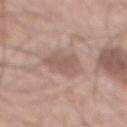A close-up tile cropped from a whole-body skin photograph, about 15 mm across. An algorithmic analysis of the crop reported a footprint of about 9.5 mm², a shape eccentricity near 0.5, and a symmetry-axis asymmetry near 0.2. And it measured a mean CIELAB color near L≈56 a*≈18 b*≈23, roughly 8 lightness units darker than nearby skin, and a normalized lesion–skin contrast near 5.5. It also reported a border-irregularity rating of about 2.5/10 and peripheral color asymmetry of about 0.5. The subject is a male aged 68 to 72. Captured under white-light illumination. Located on the abdomen.